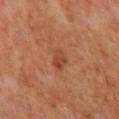This lesion was catalogued during total-body skin photography and was not selected for biopsy. The lesion is located on the mid back. Captured under cross-polarized illumination. Longest diameter approximately 3 mm. A 15 mm crop from a total-body photograph taken for skin-cancer surveillance. The subject is a male aged around 60.